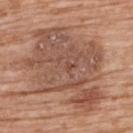lighting = white-light; diameter = ~10.5 mm (longest diameter); patient = male, about 60 years old; acquisition = total-body-photography crop, ~15 mm field of view; location = the upper back.